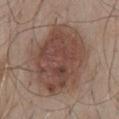Q: Is there a histopathology result?
A: total-body-photography surveillance lesion; no biopsy
Q: How was this image acquired?
A: ~15 mm crop, total-body skin-cancer survey
Q: Lesion size?
A: ~9 mm (longest diameter)
Q: What did automated image analysis measure?
A: an area of roughly 47 mm², an eccentricity of roughly 0.65, and a symmetry-axis asymmetry near 0.1; a lesion color around L≈45 a*≈18 b*≈24 in CIELAB and a lesion–skin lightness drop of about 11; a classifier nevus-likeness of about 70/100 and lesion-presence confidence of about 100/100
Q: What lighting was used for the tile?
A: white-light
Q: What are the patient's age and sex?
A: male, in their mid-50s
Q: Lesion location?
A: the mid back